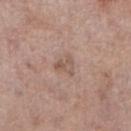body site = the right lower leg | acquisition = ~15 mm crop, total-body skin-cancer survey | tile lighting = white-light | size = about 2.5 mm | patient = female, in their mid-80s or older.